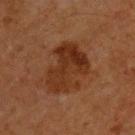subject=male, in their mid- to late 60s
lesion diameter=~6 mm (longest diameter)
tile lighting=cross-polarized illumination
TBP lesion metrics=about 8 CIELAB-L* units darker than the surrounding skin and a normalized lesion–skin contrast near 8.5
image source=15 mm crop, total-body photography
location=the upper back